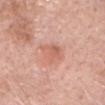No biopsy was performed on this lesion — it was imaged during a full skin examination and was not determined to be concerning. A female subject, approximately 75 years of age. Approximately 3 mm at its widest. A 15 mm close-up tile from a total-body photography series done for melanoma screening. The lesion-visualizer software estimated a mean CIELAB color near L≈62 a*≈25 b*≈30, about 8 CIELAB-L* units darker than the surrounding skin, and a lesion-to-skin contrast of about 5 (normalized; higher = more distinct). On the head or neck.